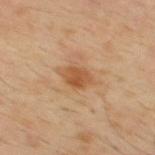{"biopsy_status": "not biopsied; imaged during a skin examination", "image": {"source": "total-body photography crop", "field_of_view_mm": 15}, "lighting": "cross-polarized", "site": "mid back", "patient": {"sex": "male", "age_approx": 30}, "lesion_size": {"long_diameter_mm_approx": 3.0}}